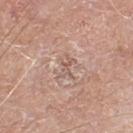Assessment: The lesion was photographed on a routine skin check and not biopsied; there is no pathology result. Background: An algorithmic analysis of the crop reported a detector confidence of about 85 out of 100 that the crop contains a lesion. A male subject, aged around 80. Measured at roughly 3 mm in maximum diameter. On the leg. Imaged with white-light lighting. This image is a 15 mm lesion crop taken from a total-body photograph.